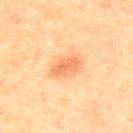Automated tile analysis of the lesion measured a lesion area of about 5 mm², a shape eccentricity near 0.85, and a shape-asymmetry score of about 0.25 (0 = symmetric). The subject is a male aged 63–67. The lesion is on the chest. A region of skin cropped from a whole-body photographic capture, roughly 15 mm wide. Imaged with cross-polarized lighting. The recorded lesion diameter is about 3.5 mm.